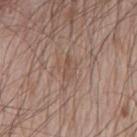workup = imaged on a skin check; not biopsied
illumination = white-light illumination
lesion size = ~2.5 mm (longest diameter)
imaging modality = total-body-photography crop, ~15 mm field of view
subject = male, aged 78 to 82
body site = the chest